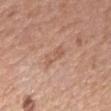Clinical impression: Captured during whole-body skin photography for melanoma surveillance; the lesion was not biopsied. Clinical summary: Captured under white-light illumination. Cropped from a whole-body photographic skin survey; the tile spans about 15 mm. The subject is a female about 60 years old. The lesion-visualizer software estimated a lesion area of about 4 mm², an outline eccentricity of about 0.95 (0 = round, 1 = elongated), and two-axis asymmetry of about 0.35. The software also gave border irregularity of about 4 on a 0–10 scale, a within-lesion color-variation index near 0.5/10, and radial color variation of about 0. And it measured a nevus-likeness score of about 0/100. The recorded lesion diameter is about 3.5 mm. From the right forearm.